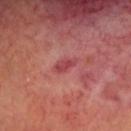notes = total-body-photography surveillance lesion; no biopsy
location = the head or neck
patient = male, about 60 years old
image source = ~15 mm crop, total-body skin-cancer survey